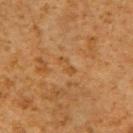Q: Was this lesion biopsied?
A: total-body-photography surveillance lesion; no biopsy
Q: Automated lesion metrics?
A: a lesion color around L≈42 a*≈19 b*≈36 in CIELAB and a lesion–skin lightness drop of about 5; a within-lesion color-variation index near 0/10 and peripheral color asymmetry of about 0
Q: What lighting was used for the tile?
A: cross-polarized illumination
Q: What kind of image is this?
A: ~15 mm tile from a whole-body skin photo
Q: Patient demographics?
A: male, roughly 60 years of age
Q: Where on the body is the lesion?
A: the right upper arm